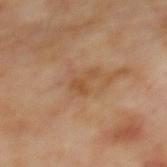Imaged during a routine full-body skin examination; the lesion was not biopsied and no histopathology is available. Automated image analysis of the tile measured a nevus-likeness score of about 0/100 and lesion-presence confidence of about 100/100. A close-up tile cropped from a whole-body skin photograph, about 15 mm across. A male patient, about 70 years old. About 2.5 mm across. The lesion is located on the mid back. This is a cross-polarized tile.